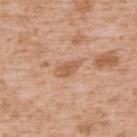biopsy_status: not biopsied; imaged during a skin examination
patient:
  sex: male
  age_approx: 65
lighting: white-light
lesion_size:
  long_diameter_mm_approx: 3.0
image:
  source: total-body photography crop
  field_of_view_mm: 15
site: upper back